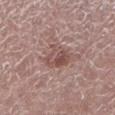<case>
  <biopsy_status>not biopsied; imaged during a skin examination</biopsy_status>
  <lesion_size>
    <long_diameter_mm_approx>4.5</long_diameter_mm_approx>
  </lesion_size>
  <lighting>white-light</lighting>
  <image>
    <source>total-body photography crop</source>
    <field_of_view_mm>15</field_of_view_mm>
  </image>
  <patient>
    <sex>male</sex>
    <age_approx>65</age_approx>
  </patient>
  <site>leg</site>
</case>